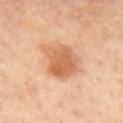The lesion was photographed on a routine skin check and not biopsied; there is no pathology result. The lesion's longest dimension is about 5.5 mm. The lesion is on the chest. The tile uses cross-polarized illumination. Cropped from a whole-body photographic skin survey; the tile spans about 15 mm. The patient is a female aged 43–47.